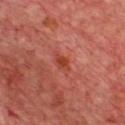Assessment: Imaged during a routine full-body skin examination; the lesion was not biopsied and no histopathology is available. Acquisition and patient details: Cropped from a whole-body photographic skin survey; the tile spans about 15 mm. Located on the chest. A male subject, aged 63 to 67. Imaged with cross-polarized lighting.